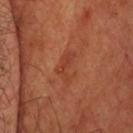notes = imaged on a skin check; not biopsied | acquisition = ~15 mm tile from a whole-body skin photo | illumination = cross-polarized illumination | site = the head or neck | patient = male, aged 63–67 | diameter = ~4 mm (longest diameter).